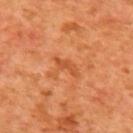A close-up tile cropped from a whole-body skin photograph, about 15 mm across. A female subject, about 40 years old. Located on the upper back.